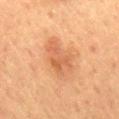Image and clinical context: This is a cross-polarized tile. On the mid back. Cropped from a total-body skin-imaging series; the visible field is about 15 mm. Automated tile analysis of the lesion measured a footprint of about 13 mm². The software also gave an automated nevus-likeness rating near 50 out of 100 and lesion-presence confidence of about 100/100. The patient is a female aged 53–57.